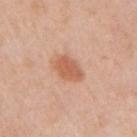biopsy_status: not biopsied; imaged during a skin examination
site: right upper arm
patient:
  sex: female
  age_approx: 40
lighting: white-light
image:
  source: total-body photography crop
  field_of_view_mm: 15
lesion_size:
  long_diameter_mm_approx: 3.5
automated_metrics:
  cielab_L: 60
  cielab_a: 24
  cielab_b: 34
  vs_skin_darker_L: 10.0
  vs_skin_contrast_norm: 7.0
  border_irregularity_0_10: 2.0
  color_variation_0_10: 2.5
  peripheral_color_asymmetry: 1.0
  lesion_detection_confidence_0_100: 100